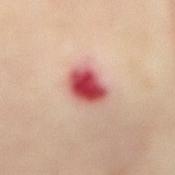Located on the abdomen.
This is a cross-polarized tile.
An algorithmic analysis of the crop reported a footprint of about 9 mm², an eccentricity of roughly 0.7, and two-axis asymmetry of about 0.2.
A roughly 15 mm field-of-view crop from a total-body skin photograph.
Longest diameter approximately 4 mm.
A female subject, aged 63 to 67.